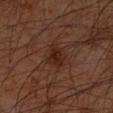  biopsy_status: not biopsied; imaged during a skin examination
  lesion_size:
    long_diameter_mm_approx: 3.5
  image:
    source: total-body photography crop
    field_of_view_mm: 15
  lighting: cross-polarized
  site: right forearm
  automated_metrics:
    area_mm2_approx: 6.5
    eccentricity: 0.7
    shape_asymmetry: 0.25
    cielab_L: 22
    cielab_a: 18
    cielab_b: 22
    vs_skin_darker_L: 6.0
    vs_skin_contrast_norm: 7.5
    border_irregularity_0_10: 2.5
    color_variation_0_10: 2.5
    peripheral_color_asymmetry: 1.0
  patient:
    sex: male
    age_approx: 60